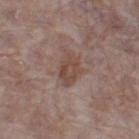{"biopsy_status": "not biopsied; imaged during a skin examination", "image": {"source": "total-body photography crop", "field_of_view_mm": 15}, "lesion_size": {"long_diameter_mm_approx": 3.5}, "patient": {"sex": "female", "age_approx": "85+"}, "automated_metrics": {"cielab_L": 46, "cielab_a": 17, "cielab_b": 23, "vs_skin_darker_L": 8.0, "border_irregularity_0_10": 2.5, "color_variation_0_10": 3.5, "nevus_likeness_0_100": 5, "lesion_detection_confidence_0_100": 100}, "site": "left thigh", "lighting": "white-light"}